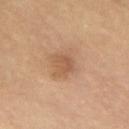| feature | finding |
|---|---|
| subject | male, aged 63 to 67 |
| imaging modality | 15 mm crop, total-body photography |
| body site | the chest |